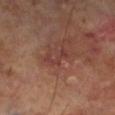Impression:
This lesion was catalogued during total-body skin photography and was not selected for biopsy.
Clinical summary:
Cropped from a total-body skin-imaging series; the visible field is about 15 mm. A male subject approximately 70 years of age. The tile uses cross-polarized illumination. The lesion is located on the left lower leg. Approximately 3 mm at its widest.